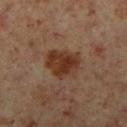Imaged during a routine full-body skin examination; the lesion was not biopsied and no histopathology is available. Cropped from a total-body skin-imaging series; the visible field is about 15 mm. This is a cross-polarized tile. About 4.5 mm across. The patient is a male approximately 60 years of age. From the right lower leg.